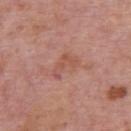  image:
    source: total-body photography crop
    field_of_view_mm: 15
  automated_metrics:
    cielab_L: 53
    cielab_a: 25
    cielab_b: 28
    vs_skin_darker_L: 7.0
  lesion_size:
    long_diameter_mm_approx: 3.5
  site: upper back
  lighting: white-light
  patient:
    sex: male
    age_approx: 75